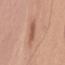This lesion was catalogued during total-body skin photography and was not selected for biopsy.
Automated image analysis of the tile measured a footprint of about 4.5 mm², a shape eccentricity near 0.35, and a symmetry-axis asymmetry near 0.2. It also reported roughly 9 lightness units darker than nearby skin and a lesion-to-skin contrast of about 6 (normalized; higher = more distinct). The software also gave a border-irregularity index near 2/10, internal color variation of about 2.5 on a 0–10 scale, and peripheral color asymmetry of about 1.
On the left upper arm.
A male subject, about 75 years old.
Longest diameter approximately 2.5 mm.
This is a white-light tile.
Cropped from a whole-body photographic skin survey; the tile spans about 15 mm.